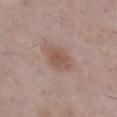This lesion was catalogued during total-body skin photography and was not selected for biopsy. This is a white-light tile. About 4 mm across. Located on the right lower leg. The subject is a female aged approximately 60. Cropped from a whole-body photographic skin survey; the tile spans about 15 mm. Automated image analysis of the tile measured a lesion color around L≈53 a*≈19 b*≈25 in CIELAB, roughly 8 lightness units darker than nearby skin, and a lesion-to-skin contrast of about 6.5 (normalized; higher = more distinct). And it measured an automated nevus-likeness rating near 80 out of 100 and a lesion-detection confidence of about 100/100.